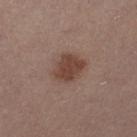follow-up — total-body-photography surveillance lesion; no biopsy
patient — female, aged 28 to 32
image — total-body-photography crop, ~15 mm field of view
diameter — ≈3.5 mm
anatomic site — the leg